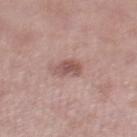Part of a total-body skin-imaging series; this lesion was reviewed on a skin check and was not flagged for biopsy. A male subject aged 53–57. A lesion tile, about 15 mm wide, cut from a 3D total-body photograph. Captured under white-light illumination. From the right lower leg. An algorithmic analysis of the crop reported an area of roughly 4 mm². The software also gave a mean CIELAB color near L≈53 a*≈21 b*≈23 and a lesion–skin lightness drop of about 11. Measured at roughly 2.5 mm in maximum diameter.